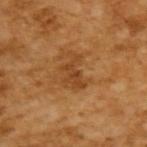The lesion was tiled from a total-body skin photograph and was not biopsied. A 15 mm close-up extracted from a 3D total-body photography capture. A male patient aged around 65.